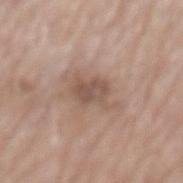The lesion was tiled from a total-body skin photograph and was not biopsied. An algorithmic analysis of the crop reported a lesion area of about 8 mm², an outline eccentricity of about 0.75 (0 = round, 1 = elongated), and two-axis asymmetry of about 0.3. The software also gave a lesion–skin lightness drop of about 9 and a normalized lesion–skin contrast near 6.5. A male patient, in their mid-70s. A lesion tile, about 15 mm wide, cut from a 3D total-body photograph. Captured under white-light illumination. Located on the back. The recorded lesion diameter is about 4.5 mm.